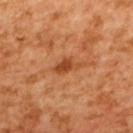No biopsy was performed on this lesion — it was imaged during a full skin examination and was not determined to be concerning. A region of skin cropped from a whole-body photographic capture, roughly 15 mm wide. A female patient, about 55 years old. Measured at roughly 3.5 mm in maximum diameter. On the upper back.